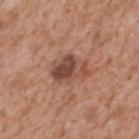Impression: The lesion was tiled from a total-body skin photograph and was not biopsied. Background: A close-up tile cropped from a whole-body skin photograph, about 15 mm across. The lesion is on the mid back. A male subject in their mid- to late 60s. Approximately 4.5 mm at its widest. An algorithmic analysis of the crop reported a shape eccentricity near 0.75. The software also gave a lesion color around L≈48 a*≈23 b*≈28 in CIELAB, about 11 CIELAB-L* units darker than the surrounding skin, and a normalized lesion–skin contrast near 8. It also reported border irregularity of about 3 on a 0–10 scale, a color-variation rating of about 7/10, and radial color variation of about 2.5. It also reported an automated nevus-likeness rating near 40 out of 100 and a lesion-detection confidence of about 100/100. The tile uses white-light illumination.